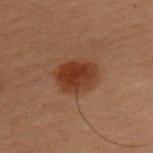Assessment:
No biopsy was performed on this lesion — it was imaged during a full skin examination and was not determined to be concerning.
Background:
A close-up tile cropped from a whole-body skin photograph, about 15 mm across. A female subject, aged 48 to 52. The lesion is located on the left upper arm.